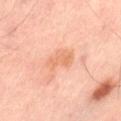| feature | finding |
|---|---|
| notes | imaged on a skin check; not biopsied |
| acquisition | ~15 mm crop, total-body skin-cancer survey |
| automated metrics | a lesion area of about 6 mm² and a symmetry-axis asymmetry near 0.3; a border-irregularity index near 3.5/10, a within-lesion color-variation index near 2/10, and peripheral color asymmetry of about 0.5; a nevus-likeness score of about 0/100 and lesion-presence confidence of about 100/100 |
| anatomic site | the leg |
| patient | male, approximately 65 years of age |